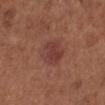Clinical impression:
Imaged during a routine full-body skin examination; the lesion was not biopsied and no histopathology is available.